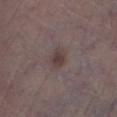Clinical impression:
Recorded during total-body skin imaging; not selected for excision or biopsy.
Clinical summary:
Located on the right lower leg. An algorithmic analysis of the crop reported a mean CIELAB color near L≈39 a*≈13 b*≈16, a lesion–skin lightness drop of about 8, and a lesion-to-skin contrast of about 7 (normalized; higher = more distinct). And it measured internal color variation of about 2 on a 0–10 scale and a peripheral color-asymmetry measure near 0.5. A roughly 15 mm field-of-view crop from a total-body skin photograph. The tile uses white-light illumination. Measured at roughly 2.5 mm in maximum diameter. The subject is a male in their 70s.